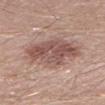Assessment:
No biopsy was performed on this lesion — it was imaged during a full skin examination and was not determined to be concerning.
Background:
The lesion is on the left lower leg. A 15 mm close-up extracted from a 3D total-body photography capture. A male patient aged around 30.